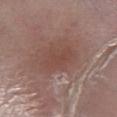* biopsy status — total-body-photography surveillance lesion; no biopsy
* lesion size — about 5 mm
* image — total-body-photography crop, ~15 mm field of view
* subject — male, aged 63 to 67
* illumination — white-light illumination
* anatomic site — the left lower leg